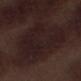{"biopsy_status": "not biopsied; imaged during a skin examination", "lighting": "white-light", "image": {"source": "total-body photography crop", "field_of_view_mm": 15}, "lesion_size": {"long_diameter_mm_approx": 9.0}, "automated_metrics": {"cielab_L": 18, "cielab_a": 14, "cielab_b": 13, "vs_skin_darker_L": 5.0, "vs_skin_contrast_norm": 7.0}, "patient": {"sex": "male", "age_approx": 70}, "site": "left lower leg"}